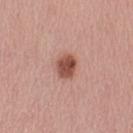<tbp_lesion>
<biopsy_status>not biopsied; imaged during a skin examination</biopsy_status>
<automated_metrics>
  <nevus_likeness_0_100>100</nevus_likeness_0_100>
  <lesion_detection_confidence_0_100>100</lesion_detection_confidence_0_100>
</automated_metrics>
<lesion_size>
  <long_diameter_mm_approx>3.0</long_diameter_mm_approx>
</lesion_size>
<site>left upper arm</site>
<image>
  <source>total-body photography crop</source>
  <field_of_view_mm>15</field_of_view_mm>
</image>
<lighting>white-light</lighting>
<patient>
  <sex>female</sex>
  <age_approx>55</age_approx>
</patient>
</tbp_lesion>